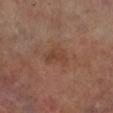{"biopsy_status": "not biopsied; imaged during a skin examination", "automated_metrics": {"eccentricity": 0.65, "shape_asymmetry": 0.45, "peripheral_color_asymmetry": 1.0, "lesion_detection_confidence_0_100": 100}, "lesion_size": {"long_diameter_mm_approx": 3.5}, "lighting": "cross-polarized", "image": {"source": "total-body photography crop", "field_of_view_mm": 15}, "site": "left lower leg", "patient": {"sex": "male", "age_approx": 70}}